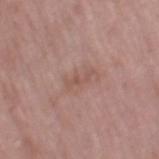Captured during whole-body skin photography for melanoma surveillance; the lesion was not biopsied.